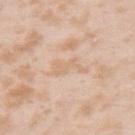Recorded during total-body skin imaging; not selected for excision or biopsy. About 4 mm across. The total-body-photography lesion software estimated a footprint of about 5 mm² and an outline eccentricity of about 0.9 (0 = round, 1 = elongated). And it measured a classifier nevus-likeness of about 0/100. Captured under white-light illumination. A female patient in their mid- to late 20s. Located on the right upper arm. Cropped from a total-body skin-imaging series; the visible field is about 15 mm.